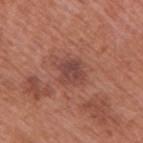The lesion is located on the right upper arm. A roughly 15 mm field-of-view crop from a total-body skin photograph. A male subject aged approximately 70. Automated image analysis of the tile measured a lesion area of about 5.5 mm², a shape eccentricity near 0.3, and a symmetry-axis asymmetry near 0.2. The analysis additionally found a border-irregularity rating of about 2.5/10, a color-variation rating of about 2.5/10, and radial color variation of about 1. This is a white-light tile.